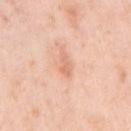Approximately 2.5 mm at its widest. A female patient, in their mid- to late 60s. On the arm. This image is a 15 mm lesion crop taken from a total-body photograph.